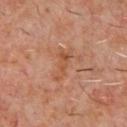The lesion was tiled from a total-body skin photograph and was not biopsied. Located on the chest. The lesion's longest dimension is about 4.5 mm. A male patient aged around 60. A lesion tile, about 15 mm wide, cut from a 3D total-body photograph.